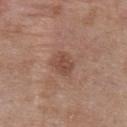Notes:
• follow-up — imaged on a skin check; not biopsied
• subject — female, aged around 40
• illumination — white-light illumination
• lesion size — about 3 mm
• location — the back
• imaging modality — total-body-photography crop, ~15 mm field of view